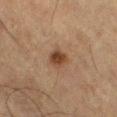biopsy status=no biopsy performed (imaged during a skin exam)
image=total-body-photography crop, ~15 mm field of view
patient=male, aged 73–77
anatomic site=the left thigh